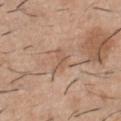On the chest.
This is a white-light tile.
An algorithmic analysis of the crop reported a footprint of about 4 mm², a shape eccentricity near 0.85, and a symmetry-axis asymmetry near 0.45. And it measured an average lesion color of about L≈57 a*≈18 b*≈30 (CIELAB), a lesion–skin lightness drop of about 7, and a normalized border contrast of about 5.
A close-up tile cropped from a whole-body skin photograph, about 15 mm across.
A male patient aged 38 to 42.